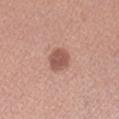{"biopsy_status": "not biopsied; imaged during a skin examination", "automated_metrics": {"area_mm2_approx": 6.5, "eccentricity": 0.3, "shape_asymmetry": 0.15, "cielab_L": 54, "cielab_a": 23, "cielab_b": 26, "vs_skin_contrast_norm": 8.0, "border_irregularity_0_10": 1.5, "color_variation_0_10": 2.0, "peripheral_color_asymmetry": 0.5, "nevus_likeness_0_100": 65, "lesion_detection_confidence_0_100": 100}, "image": {"source": "total-body photography crop", "field_of_view_mm": 15}, "patient": {"sex": "female", "age_approx": 40}, "lighting": "white-light", "site": "left forearm"}